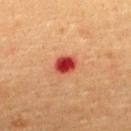No biopsy was performed on this lesion — it was imaged during a full skin examination and was not determined to be concerning. Imaged with cross-polarized lighting. Longest diameter approximately 2.5 mm. The total-body-photography lesion software estimated a mean CIELAB color near L≈36 a*≈35 b*≈30, roughly 16 lightness units darker than nearby skin, and a normalized border contrast of about 12.5. It also reported an automated nevus-likeness rating near 0 out of 100. A female patient, in their mid-50s. A 15 mm close-up tile from a total-body photography series done for melanoma screening. The lesion is located on the upper back.